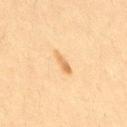The lesion was tiled from a total-body skin photograph and was not biopsied. A female patient, aged 38–42. An algorithmic analysis of the crop reported an area of roughly 3 mm², a shape eccentricity near 0.95, and a shape-asymmetry score of about 0.25 (0 = symmetric). And it measured a border-irregularity index near 3/10, a within-lesion color-variation index near 0.5/10, and radial color variation of about 0. About 3 mm across. A 15 mm crop from a total-body photograph taken for skin-cancer surveillance. Imaged with cross-polarized lighting. Located on the leg.